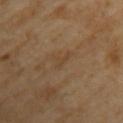notes — imaged on a skin check; not biopsied
patient — female, about 70 years old
image source — ~15 mm crop, total-body skin-cancer survey
illumination — cross-polarized illumination
lesion size — ~2.5 mm (longest diameter)
anatomic site — the left upper arm